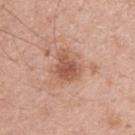Part of a total-body skin-imaging series; this lesion was reviewed on a skin check and was not flagged for biopsy.